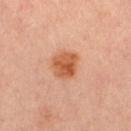| feature | finding |
|---|---|
| workup | no biopsy performed (imaged during a skin exam) |
| patient | female, roughly 35 years of age |
| location | the left thigh |
| image | total-body-photography crop, ~15 mm field of view |